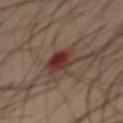| feature | finding |
|---|---|
| biopsy status | imaged on a skin check; not biopsied |
| location | the abdomen |
| subject | male, about 60 years old |
| image source | 15 mm crop, total-body photography |
| lesion size | about 4 mm |
| TBP lesion metrics | a mean CIELAB color near L≈33 a*≈21 b*≈22, about 10 CIELAB-L* units darker than the surrounding skin, and a normalized lesion–skin contrast near 9; an automated nevus-likeness rating near 0 out of 100 and a detector confidence of about 100 out of 100 that the crop contains a lesion |
| tile lighting | cross-polarized illumination |